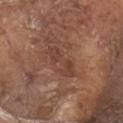Imaged during a routine full-body skin examination; the lesion was not biopsied and no histopathology is available.
Approximately 4.5 mm at its widest.
A lesion tile, about 15 mm wide, cut from a 3D total-body photograph.
The lesion is on the head or neck.
A male subject, aged 78–82.